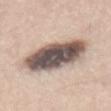Q: What is the anatomic site?
A: the lower back
Q: What are the patient's age and sex?
A: male, aged around 60
Q: What kind of image is this?
A: 15 mm crop, total-body photography
Q: What lighting was used for the tile?
A: white-light